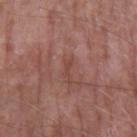The lesion was photographed on a routine skin check and not biopsied; there is no pathology result.
Cropped from a total-body skin-imaging series; the visible field is about 15 mm.
The lesion is located on the right upper arm.
The subject is a male about 65 years old.
This is a white-light tile.
About 2 mm across.